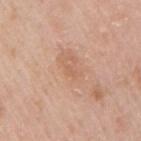No biopsy was performed on this lesion — it was imaged during a full skin examination and was not determined to be concerning. Longest diameter approximately 1 mm. This is a white-light tile. A 15 mm crop from a total-body photograph taken for skin-cancer surveillance. Automated image analysis of the tile measured a lesion area of about 0.5 mm² and a shape eccentricity near 0.9. The software also gave an average lesion color of about L≈60 a*≈22 b*≈32 (CIELAB), roughly 5 lightness units darker than nearby skin, and a lesion-to-skin contrast of about 3.5 (normalized; higher = more distinct). The software also gave a border-irregularity index near 4/10 and peripheral color asymmetry of about 0. And it measured a nevus-likeness score of about 0/100 and lesion-presence confidence of about 100/100. A male patient, in their mid- to late 30s. On the right upper arm.